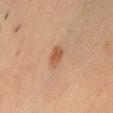The lesion was photographed on a routine skin check and not biopsied; there is no pathology result.
This is a cross-polarized tile.
The recorded lesion diameter is about 3 mm.
Located on the chest.
A 15 mm close-up tile from a total-body photography series done for melanoma screening.
An algorithmic analysis of the crop reported a border-irregularity rating of about 2/10, a within-lesion color-variation index near 2.5/10, and peripheral color asymmetry of about 1. It also reported an automated nevus-likeness rating near 85 out of 100 and lesion-presence confidence of about 100/100.
A male subject, aged around 35.